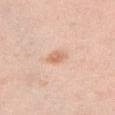Imaged during a routine full-body skin examination; the lesion was not biopsied and no histopathology is available. The patient is a female approximately 40 years of age. The lesion is located on the abdomen. Longest diameter approximately 2.5 mm. A roughly 15 mm field-of-view crop from a total-body skin photograph. The total-body-photography lesion software estimated a nevus-likeness score of about 70/100 and lesion-presence confidence of about 100/100.